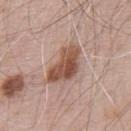Q: Is there a histopathology result?
A: catalogued during a skin exam; not biopsied
Q: What are the patient's age and sex?
A: male, roughly 70 years of age
Q: How was this image acquired?
A: total-body-photography crop, ~15 mm field of view
Q: What is the anatomic site?
A: the upper back
Q: How large is the lesion?
A: about 5.5 mm
Q: What lighting was used for the tile?
A: white-light